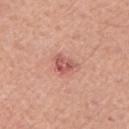Imaged during a routine full-body skin examination; the lesion was not biopsied and no histopathology is available.
The total-body-photography lesion software estimated border irregularity of about 3.5 on a 0–10 scale, internal color variation of about 3.5 on a 0–10 scale, and radial color variation of about 1. It also reported a nevus-likeness score of about 10/100 and a detector confidence of about 100 out of 100 that the crop contains a lesion.
Captured under white-light illumination.
A male patient in their mid- to late 50s.
A region of skin cropped from a whole-body photographic capture, roughly 15 mm wide.
Longest diameter approximately 3 mm.
The lesion is located on the left upper arm.